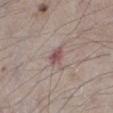biopsy status: catalogued during a skin exam; not biopsied | location: the left lower leg | patient: male, aged approximately 75 | image-analysis metrics: an outline eccentricity of about 0.75 (0 = round, 1 = elongated); an automated nevus-likeness rating near 0 out of 100 and a lesion-detection confidence of about 100/100 | acquisition: 15 mm crop, total-body photography | size: ≈2.5 mm | illumination: white-light illumination.